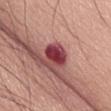notes: imaged on a skin check; not biopsied | subject: male, aged approximately 70 | image source: total-body-photography crop, ~15 mm field of view | location: the abdomen | image-analysis metrics: a footprint of about 6.5 mm², an eccentricity of roughly 0.65, and a symmetry-axis asymmetry near 0.25; an average lesion color of about L≈40 a*≈35 b*≈20 (CIELAB), roughly 18 lightness units darker than nearby skin, and a lesion-to-skin contrast of about 13.5 (normalized; higher = more distinct) | lesion diameter: ~3 mm (longest diameter) | tile lighting: white-light illumination.